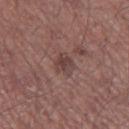Findings:
• biopsy status: catalogued during a skin exam; not biopsied
• patient: male, in their mid-50s
• body site: the left thigh
• automated lesion analysis: an automated nevus-likeness rating near 0 out of 100 and a detector confidence of about 100 out of 100 that the crop contains a lesion
• imaging modality: ~15 mm crop, total-body skin-cancer survey